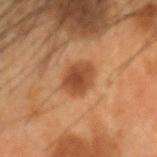Impression:
This lesion was catalogued during total-body skin photography and was not selected for biopsy.
Image and clinical context:
Captured under cross-polarized illumination. A close-up tile cropped from a whole-body skin photograph, about 15 mm across. A male subject in their mid-50s. The lesion is on the head or neck.